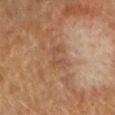Q: Was this lesion biopsied?
A: no biopsy performed (imaged during a skin exam)
Q: How was this image acquired?
A: ~15 mm crop, total-body skin-cancer survey
Q: Lesion location?
A: the right forearm
Q: Patient demographics?
A: female, aged approximately 55